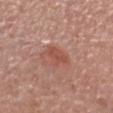  biopsy_status: not biopsied; imaged during a skin examination
  site: head or neck
  image:
    source: total-body photography crop
    field_of_view_mm: 15
  patient:
    sex: male
    age_approx: 45
  automated_metrics:
    vs_skin_darker_L: 8.0
    vs_skin_contrast_norm: 6.5
    color_variation_0_10: 1.5
    peripheral_color_asymmetry: 0.5
  lesion_size:
    long_diameter_mm_approx: 2.5
  lighting: white-light